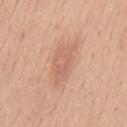biopsy_status: not biopsied; imaged during a skin examination
image:
  source: total-body photography crop
  field_of_view_mm: 15
site: mid back
patient:
  sex: male
  age_approx: 40